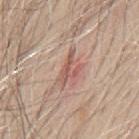Q: Was this lesion biopsied?
A: catalogued during a skin exam; not biopsied
Q: What are the patient's age and sex?
A: male, aged 63 to 67
Q: What kind of image is this?
A: 15 mm crop, total-body photography
Q: Automated lesion metrics?
A: border irregularity of about 9.5 on a 0–10 scale and radial color variation of about 0; a nevus-likeness score of about 0/100
Q: What is the anatomic site?
A: the back